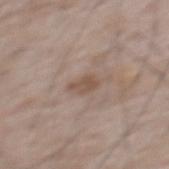Impression: No biopsy was performed on this lesion — it was imaged during a full skin examination and was not determined to be concerning. Acquisition and patient details: The total-body-photography lesion software estimated a lesion area of about 4 mm², an outline eccentricity of about 0.8 (0 = round, 1 = elongated), and a shape-asymmetry score of about 0.3 (0 = symmetric). The analysis additionally found a border-irregularity rating of about 3/10 and a peripheral color-asymmetry measure near 0.5. A close-up tile cropped from a whole-body skin photograph, about 15 mm across. A male patient, roughly 65 years of age. The lesion is located on the mid back.